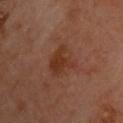No biopsy was performed on this lesion — it was imaged during a full skin examination and was not determined to be concerning.
A region of skin cropped from a whole-body photographic capture, roughly 15 mm wide.
The patient is a male aged approximately 65.
The tile uses cross-polarized illumination.
From the upper back.
Longest diameter approximately 4 mm.